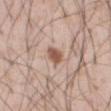workup = no biopsy performed (imaged during a skin exam); subject = male, aged around 55; anatomic site = the abdomen; image source = ~15 mm tile from a whole-body skin photo; lighting = white-light.